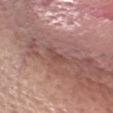Q: Was this lesion biopsied?
A: imaged on a skin check; not biopsied
Q: What is the anatomic site?
A: the head or neck
Q: How was this image acquired?
A: ~15 mm tile from a whole-body skin photo
Q: Who is the patient?
A: female, aged around 65